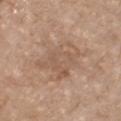No biopsy was performed on this lesion — it was imaged during a full skin examination and was not determined to be concerning. A male patient, aged 68 to 72. Automated image analysis of the tile measured a lesion color around L≈56 a*≈17 b*≈29 in CIELAB, a lesion–skin lightness drop of about 7, and a normalized lesion–skin contrast near 5. It also reported a border-irregularity rating of about 3.5/10 and a color-variation rating of about 3.5/10. Cropped from a total-body skin-imaging series; the visible field is about 15 mm. Located on the front of the torso. Measured at roughly 5.5 mm in maximum diameter. Captured under white-light illumination.